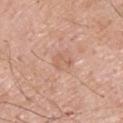No biopsy was performed on this lesion — it was imaged during a full skin examination and was not determined to be concerning. Imaged with white-light lighting. Automated tile analysis of the lesion measured an area of roughly 3.5 mm², an outline eccentricity of about 0.7 (0 = round, 1 = elongated), and a shape-asymmetry score of about 0.45 (0 = symmetric). The analysis additionally found a lesion color around L≈61 a*≈21 b*≈30 in CIELAB. And it measured a nevus-likeness score of about 0/100 and a detector confidence of about 100 out of 100 that the crop contains a lesion. Approximately 2.5 mm at its widest. A male subject, aged 58–62. The lesion is located on the upper back. Cropped from a total-body skin-imaging series; the visible field is about 15 mm.